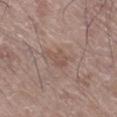{
  "image": {
    "source": "total-body photography crop",
    "field_of_view_mm": 15
  },
  "site": "right lower leg",
  "patient": {
    "sex": "male",
    "age_approx": 65
  },
  "lesion_size": {
    "long_diameter_mm_approx": 4.0
  },
  "automated_metrics": {
    "area_mm2_approx": 7.5,
    "eccentricity": 0.8,
    "shape_asymmetry": 0.4,
    "vs_skin_darker_L": 6.0,
    "vs_skin_contrast_norm": 5.0,
    "border_irregularity_0_10": 5.0,
    "color_variation_0_10": 2.0,
    "peripheral_color_asymmetry": 0.5
  },
  "lighting": "white-light"
}